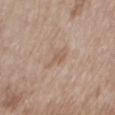Captured during whole-body skin photography for melanoma surveillance; the lesion was not biopsied. A roughly 15 mm field-of-view crop from a total-body skin photograph. A female patient, aged approximately 75. The lesion is on the mid back. Measured at roughly 3.5 mm in maximum diameter. Imaged with white-light lighting. Automated tile analysis of the lesion measured a detector confidence of about 100 out of 100 that the crop contains a lesion.